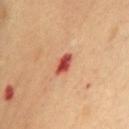site: the chest; acquisition: ~15 mm tile from a whole-body skin photo; tile lighting: cross-polarized; diameter: ≈2.5 mm; patient: female, approximately 65 years of age.